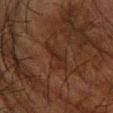Imaged during a routine full-body skin examination; the lesion was not biopsied and no histopathology is available. The subject is a male about 65 years old. The lesion is located on the right forearm. Cropped from a total-body skin-imaging series; the visible field is about 15 mm. Captured under cross-polarized illumination.